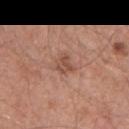No biopsy was performed on this lesion — it was imaged during a full skin examination and was not determined to be concerning.
On the back.
The tile uses white-light illumination.
The recorded lesion diameter is about 2.5 mm.
A male patient, roughly 65 years of age.
A 15 mm close-up tile from a total-body photography series done for melanoma screening.